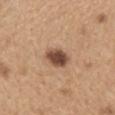Captured during whole-body skin photography for melanoma surveillance; the lesion was not biopsied.
The lesion is located on the upper back.
The subject is a female aged 58–62.
A 15 mm crop from a total-body photograph taken for skin-cancer surveillance.